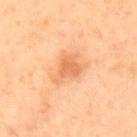Part of a total-body skin-imaging series; this lesion was reviewed on a skin check and was not flagged for biopsy. The lesion's longest dimension is about 4 mm. A region of skin cropped from a whole-body photographic capture, roughly 15 mm wide. A male subject approximately 65 years of age. From the back. The tile uses cross-polarized illumination.